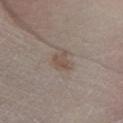{
  "biopsy_status": "not biopsied; imaged during a skin examination",
  "image": {
    "source": "total-body photography crop",
    "field_of_view_mm": 15
  },
  "automated_metrics": {
    "cielab_L": 50,
    "cielab_a": 13,
    "cielab_b": 22,
    "vs_skin_darker_L": 7.0,
    "vs_skin_contrast_norm": 6.0,
    "peripheral_color_asymmetry": 1.0,
    "nevus_likeness_0_100": 30,
    "lesion_detection_confidence_0_100": 100
  },
  "patient": {
    "sex": "male",
    "age_approx": 75
  },
  "site": "right lower leg"
}